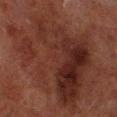workup: imaged on a skin check; not biopsied | imaging modality: 15 mm crop, total-body photography | tile lighting: cross-polarized | patient: male, in their 70s | anatomic site: the left lower leg.